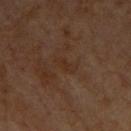Notes:
• biopsy status · imaged on a skin check; not biopsied
• image source · ~15 mm crop, total-body skin-cancer survey
• patient · male, in their 60s
• TBP lesion metrics · an area of roughly 2.5 mm², an outline eccentricity of about 0.9 (0 = round, 1 = elongated), and two-axis asymmetry of about 0.3; a lesion color around L≈26 a*≈16 b*≈24 in CIELAB, roughly 3 lightness units darker than nearby skin, and a normalized lesion–skin contrast near 5
• body site · the chest